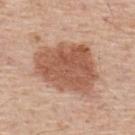This lesion was catalogued during total-body skin photography and was not selected for biopsy.
The total-body-photography lesion software estimated an area of roughly 32 mm², a shape eccentricity near 0.7, and a shape-asymmetry score of about 0.2 (0 = symmetric). The software also gave a lesion color around L≈56 a*≈23 b*≈31 in CIELAB, about 13 CIELAB-L* units darker than the surrounding skin, and a normalized lesion–skin contrast near 8.5.
About 7.5 mm across.
The patient is a male roughly 55 years of age.
Located on the upper back.
Captured under white-light illumination.
This image is a 15 mm lesion crop taken from a total-body photograph.